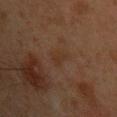Q: Was a biopsy performed?
A: imaged on a skin check; not biopsied
Q: Patient demographics?
A: male, aged 43–47
Q: What is the lesion's diameter?
A: ~2.5 mm (longest diameter)
Q: Automated lesion metrics?
A: a footprint of about 3.5 mm², an outline eccentricity of about 0.75 (0 = round, 1 = elongated), and two-axis asymmetry of about 0.3; a mean CIELAB color near L≈26 a*≈15 b*≈23 and a lesion-to-skin contrast of about 5 (normalized; higher = more distinct); border irregularity of about 2.5 on a 0–10 scale and a color-variation rating of about 1/10; a classifier nevus-likeness of about 0/100 and a detector confidence of about 100 out of 100 that the crop contains a lesion
Q: Where on the body is the lesion?
A: the left upper arm
Q: How was this image acquired?
A: ~15 mm tile from a whole-body skin photo